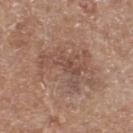The lesion was tiled from a total-body skin photograph and was not biopsied.
Located on the right thigh.
A lesion tile, about 15 mm wide, cut from a 3D total-body photograph.
The patient is a female aged approximately 75.